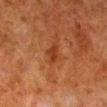biopsy_status: not biopsied; imaged during a skin examination
lighting: cross-polarized
patient:
  sex: male
  age_approx: 80
lesion_size:
  long_diameter_mm_approx: 3.0
image:
  source: total-body photography crop
  field_of_view_mm: 15
site: right lower leg
automated_metrics:
  area_mm2_approx: 4.0
  eccentricity: 0.75
  shape_asymmetry: 0.4
  cielab_L: 31
  cielab_a: 23
  cielab_b: 31
  vs_skin_darker_L: 7.0
  vs_skin_contrast_norm: 6.5
  border_irregularity_0_10: 4.0
  color_variation_0_10: 1.0
  peripheral_color_asymmetry: 0.5
  nevus_likeness_0_100: 30
  lesion_detection_confidence_0_100: 100